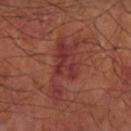Findings:
- workup: no biopsy performed (imaged during a skin exam)
- location: the right forearm
- patient: male, approximately 65 years of age
- image-analysis metrics: an average lesion color of about L≈34 a*≈27 b*≈24 (CIELAB) and roughly 6 lightness units darker than nearby skin; an automated nevus-likeness rating near 0 out of 100 and a detector confidence of about 95 out of 100 that the crop contains a lesion
- image source: ~15 mm crop, total-body skin-cancer survey
- size: about 8 mm